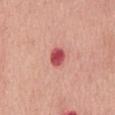Captured during whole-body skin photography for melanoma surveillance; the lesion was not biopsied. A lesion tile, about 15 mm wide, cut from a 3D total-body photograph. The lesion's longest dimension is about 2.5 mm. From the abdomen. The subject is a male about 70 years old. The tile uses white-light illumination.